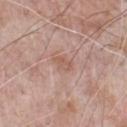Q: Was this lesion biopsied?
A: catalogued during a skin exam; not biopsied
Q: What is the anatomic site?
A: the chest
Q: Patient demographics?
A: male, aged 68 to 72
Q: How was the tile lit?
A: white-light illumination
Q: What kind of image is this?
A: 15 mm crop, total-body photography
Q: What is the lesion's diameter?
A: about 2.5 mm